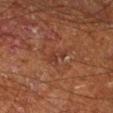<tbp_lesion>
<biopsy_status>not biopsied; imaged during a skin examination</biopsy_status>
<site>right lower leg</site>
<patient>
  <sex>male</sex>
  <age_approx>65</age_approx>
</patient>
<image>
  <source>total-body photography crop</source>
  <field_of_view_mm>15</field_of_view_mm>
</image>
<lighting>cross-polarized</lighting>
<lesion_size>
  <long_diameter_mm_approx>3.0</long_diameter_mm_approx>
</lesion_size>
<automated_metrics>
  <area_mm2_approx>2.5</area_mm2_approx>
  <eccentricity>0.9</eccentricity>
  <shape_asymmetry>0.4</shape_asymmetry>
  <vs_skin_contrast_norm>5.5</vs_skin_contrast_norm>
  <nevus_likeness_0_100>0</nevus_likeness_0_100>
  <lesion_detection_confidence_0_100>90</lesion_detection_confidence_0_100>
</automated_metrics>
</tbp_lesion>